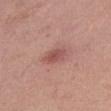Case summary:
- acquisition: total-body-photography crop, ~15 mm field of view
- body site: the right thigh
- patient: female, in their 40s